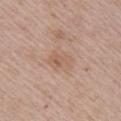Captured during whole-body skin photography for melanoma surveillance; the lesion was not biopsied. An algorithmic analysis of the crop reported an average lesion color of about L≈58 a*≈19 b*≈29 (CIELAB), roughly 6 lightness units darker than nearby skin, and a lesion-to-skin contrast of about 5 (normalized; higher = more distinct). It also reported a detector confidence of about 100 out of 100 that the crop contains a lesion. The lesion's longest dimension is about 3 mm. The lesion is located on the chest. Imaged with white-light lighting. A male subject, aged around 65. This image is a 15 mm lesion crop taken from a total-body photograph.